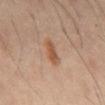follow-up: catalogued during a skin exam; not biopsied | lighting: cross-polarized | image-analysis metrics: border irregularity of about 2 on a 0–10 scale, a color-variation rating of about 2/10, and radial color variation of about 0.5; an automated nevus-likeness rating near 65 out of 100 and a lesion-detection confidence of about 100/100 | lesion diameter: about 3 mm | image source: total-body-photography crop, ~15 mm field of view | patient: male, in their mid-40s | site: the mid back.